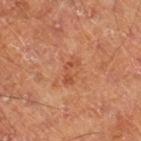follow-up — total-body-photography surveillance lesion; no biopsy | diameter — about 3 mm | subject — male, aged approximately 65 | body site — the leg | TBP lesion metrics — a lesion area of about 3.5 mm², a shape eccentricity near 0.9, and a symmetry-axis asymmetry near 0.45; a border-irregularity rating of about 5/10, a within-lesion color-variation index near 0/10, and radial color variation of about 0; an automated nevus-likeness rating near 5 out of 100 | image — total-body-photography crop, ~15 mm field of view | tile lighting — cross-polarized.